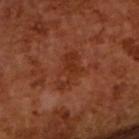{"biopsy_status": "not biopsied; imaged during a skin examination", "lesion_size": {"long_diameter_mm_approx": 4.5}, "image": {"source": "total-body photography crop", "field_of_view_mm": 15}, "lighting": "cross-polarized", "patient": {"sex": "male", "age_approx": 65}, "automated_metrics": {"color_variation_0_10": 4.5, "peripheral_color_asymmetry": 1.5, "nevus_likeness_0_100": 0, "lesion_detection_confidence_0_100": 100}}